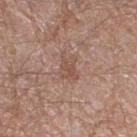Findings:
– biopsy status: imaged on a skin check; not biopsied
– subject: male, in their mid- to late 60s
– lesion size: ≈3 mm
– body site: the leg
– illumination: white-light illumination
– imaging modality: ~15 mm crop, total-body skin-cancer survey